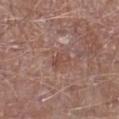Notes:
- follow-up · no biopsy performed (imaged during a skin exam)
- patient · male, about 70 years old
- illumination · white-light illumination
- image · ~15 mm crop, total-body skin-cancer survey
- location · the leg